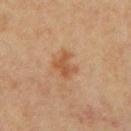subject — male, in their mid-60s
image source — 15 mm crop, total-body photography
lesion size — ~3 mm (longest diameter)
illumination — cross-polarized illumination
body site — the chest